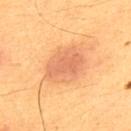The recorded lesion diameter is about 5 mm. A roughly 15 mm field-of-view crop from a total-body skin photograph. A male subject approximately 65 years of age. From the upper back. Automated tile analysis of the lesion measured about 9 CIELAB-L* units darker than the surrounding skin and a lesion-to-skin contrast of about 6 (normalized; higher = more distinct). The software also gave a nevus-likeness score of about 90/100. Imaged with cross-polarized lighting.